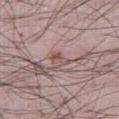  biopsy_status: not biopsied; imaged during a skin examination
  site: right thigh
  automated_metrics:
    area_mm2_approx: 3.5
    eccentricity: 0.85
    shape_asymmetry: 0.45
    lesion_detection_confidence_0_100: 95
  image:
    source: total-body photography crop
    field_of_view_mm: 15
  lesion_size:
    long_diameter_mm_approx: 3.0
  patient:
    sex: male
    age_approx: 40